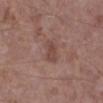Cropped from a total-body skin-imaging series; the visible field is about 15 mm.
The subject is a male aged 68 to 72.
This is a white-light tile.
The total-body-photography lesion software estimated a lesion color around L≈45 a*≈19 b*≈24 in CIELAB, about 7 CIELAB-L* units darker than the surrounding skin, and a normalized lesion–skin contrast near 6. The analysis additionally found a classifier nevus-likeness of about 0/100 and lesion-presence confidence of about 100/100.
Approximately 3 mm at its widest.
The lesion is located on the right lower leg.